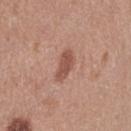workup: catalogued during a skin exam; not biopsied
site: the right thigh
image source: 15 mm crop, total-body photography
subject: female, aged 38–42